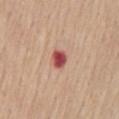No biopsy was performed on this lesion — it was imaged during a full skin examination and was not determined to be concerning.
This is a white-light tile.
A female patient aged 53 to 57.
Automated image analysis of the tile measured an area of roughly 4 mm². The software also gave a mean CIELAB color near L≈51 a*≈33 b*≈25, roughly 17 lightness units darker than nearby skin, and a lesion-to-skin contrast of about 11.5 (normalized; higher = more distinct). It also reported a border-irregularity index near 1.5/10, a color-variation rating of about 4.5/10, and radial color variation of about 1.5.
The lesion is located on the mid back.
A 15 mm crop from a total-body photograph taken for skin-cancer surveillance.
About 2.5 mm across.